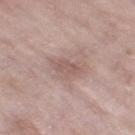The lesion was tiled from a total-body skin photograph and was not biopsied. The lesion is on the right thigh. Automated image analysis of the tile measured a lesion color around L≈56 a*≈18 b*≈22 in CIELAB and a normalized border contrast of about 5.5. The analysis additionally found border irregularity of about 2 on a 0–10 scale, internal color variation of about 1.5 on a 0–10 scale, and radial color variation of about 0.5. This is a white-light tile. A 15 mm close-up tile from a total-body photography series done for melanoma screening. A female subject approximately 70 years of age.